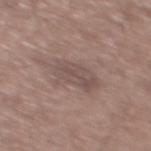Assessment: The lesion was tiled from a total-body skin photograph and was not biopsied. Image and clinical context: Longest diameter approximately 4.5 mm. A male patient, approximately 60 years of age. Automated image analysis of the tile measured a classifier nevus-likeness of about 0/100. Located on the upper back. This is a white-light tile. This image is a 15 mm lesion crop taken from a total-body photograph.